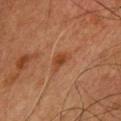– workup · catalogued during a skin exam; not biopsied
– anatomic site · the chest
– lighting · cross-polarized illumination
– acquisition · ~15 mm tile from a whole-body skin photo
– patient · male, roughly 60 years of age